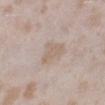Assessment:
No biopsy was performed on this lesion — it was imaged during a full skin examination and was not determined to be concerning.
Clinical summary:
The total-body-photography lesion software estimated an area of roughly 5.5 mm², a shape eccentricity near 0.8, and a symmetry-axis asymmetry near 0.45. The software also gave a mean CIELAB color near L≈62 a*≈12 b*≈26 and a lesion-to-skin contrast of about 5.5 (normalized; higher = more distinct). On the left lower leg. A female patient in their mid- to late 20s. Approximately 3.5 mm at its widest. This image is a 15 mm lesion crop taken from a total-body photograph.